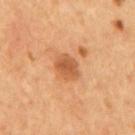No biopsy was performed on this lesion — it was imaged during a full skin examination and was not determined to be concerning. A lesion tile, about 15 mm wide, cut from a 3D total-body photograph. From the mid back. Imaged with cross-polarized lighting. A male patient, roughly 55 years of age.